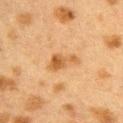The lesion was photographed on a routine skin check and not biopsied; there is no pathology result.
Cropped from a total-body skin-imaging series; the visible field is about 15 mm.
A female patient, aged 38–42.
On the upper back.